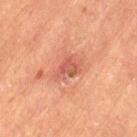Automated tile analysis of the lesion measured a footprint of about 5.5 mm², an eccentricity of roughly 0.75, and two-axis asymmetry of about 0.35. It also reported a border-irregularity index near 4/10, internal color variation of about 4 on a 0–10 scale, and a peripheral color-asymmetry measure near 1.5. A lesion tile, about 15 mm wide, cut from a 3D total-body photograph. About 3.5 mm across. Captured under cross-polarized illumination. The subject is a male in their mid-80s or older. The lesion is located on the left thigh.